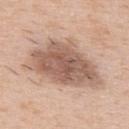Recorded during total-body skin imaging; not selected for excision or biopsy. Imaged with white-light lighting. From the upper back. A 15 mm close-up extracted from a 3D total-body photography capture. A male subject approximately 50 years of age. The lesion's longest dimension is about 9 mm. The lesion-visualizer software estimated border irregularity of about 3 on a 0–10 scale, a color-variation rating of about 4.5/10, and a peripheral color-asymmetry measure near 1.5. The analysis additionally found a lesion-detection confidence of about 100/100.